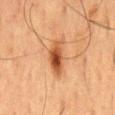{"biopsy_status": "not biopsied; imaged during a skin examination", "patient": {"sex": "male", "age_approx": 60}, "image": {"source": "total-body photography crop", "field_of_view_mm": 15}, "site": "back"}